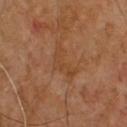No biopsy was performed on this lesion — it was imaged during a full skin examination and was not determined to be concerning. A 15 mm close-up extracted from a 3D total-body photography capture. Imaged with cross-polarized lighting. A male subject, aged 63–67.